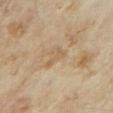The lesion was tiled from a total-body skin photograph and was not biopsied.
This is a cross-polarized tile.
A female patient, about 35 years old.
This image is a 15 mm lesion crop taken from a total-body photograph.
About 2.5 mm across.
Located on the right forearm.